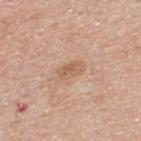biopsy status: no biopsy performed (imaged during a skin exam)
body site: the upper back
image source: ~15 mm tile from a whole-body skin photo
subject: male, aged 33 to 37
automated metrics: a border-irregularity rating of about 2/10
size: ~3 mm (longest diameter)